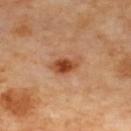<tbp_lesion>
  <biopsy_status>not biopsied; imaged during a skin examination</biopsy_status>
  <lesion_size>
    <long_diameter_mm_approx>3.5</long_diameter_mm_approx>
  </lesion_size>
  <site>upper back</site>
  <lighting>cross-polarized</lighting>
  <image>
    <source>total-body photography crop</source>
    <field_of_view_mm>15</field_of_view_mm>
  </image>
</tbp_lesion>